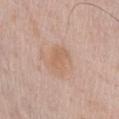Imaged during a routine full-body skin examination; the lesion was not biopsied and no histopathology is available.
The total-body-photography lesion software estimated an average lesion color of about L≈62 a*≈19 b*≈31 (CIELAB) and roughly 6 lightness units darker than nearby skin. The software also gave a nevus-likeness score of about 5/100.
Cropped from a whole-body photographic skin survey; the tile spans about 15 mm.
Located on the chest.
The tile uses white-light illumination.
The recorded lesion diameter is about 3.5 mm.
A male patient aged 68–72.